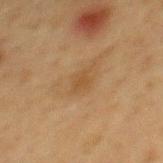Q: Was this lesion biopsied?
A: imaged on a skin check; not biopsied
Q: How was the tile lit?
A: cross-polarized illumination
Q: Where on the body is the lesion?
A: the mid back
Q: Automated lesion metrics?
A: a shape eccentricity near 0.7 and a shape-asymmetry score of about 0.25 (0 = symmetric); a lesion–skin lightness drop of about 5 and a normalized lesion–skin contrast near 5.5; border irregularity of about 2.5 on a 0–10 scale, internal color variation of about 1 on a 0–10 scale, and a peripheral color-asymmetry measure near 0.5; an automated nevus-likeness rating near 5 out of 100 and a lesion-detection confidence of about 100/100
Q: What are the patient's age and sex?
A: male, in their mid-70s
Q: What is the imaging modality?
A: ~15 mm crop, total-body skin-cancer survey
Q: How large is the lesion?
A: about 2.5 mm